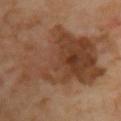Q: Is there a histopathology result?
A: total-body-photography surveillance lesion; no biopsy
Q: Automated lesion metrics?
A: an outline eccentricity of about 0.8 (0 = round, 1 = elongated) and two-axis asymmetry of about 0.3; about 9 CIELAB-L* units darker than the surrounding skin
Q: Patient demographics?
A: female, aged around 50
Q: Lesion location?
A: the chest
Q: How was this image acquired?
A: total-body-photography crop, ~15 mm field of view
Q: Lesion size?
A: ~13.5 mm (longest diameter)
Q: How was the tile lit?
A: cross-polarized